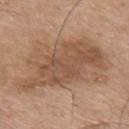{
  "biopsy_status": "not biopsied; imaged during a skin examination",
  "image": {
    "source": "total-body photography crop",
    "field_of_view_mm": 15
  },
  "patient": {
    "sex": "male",
    "age_approx": 75
  },
  "site": "mid back",
  "lesion_size": {
    "long_diameter_mm_approx": 11.0
  },
  "automated_metrics": {
    "shape_asymmetry": 0.3
  },
  "lighting": "white-light"
}